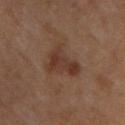biopsy_status: not biopsied; imaged during a skin examination
image:
  source: total-body photography crop
  field_of_view_mm: 15
patient:
  sex: male
  age_approx: 55
automated_metrics:
  cielab_L: 33
  cielab_a: 17
  cielab_b: 24
lesion_size:
  long_diameter_mm_approx: 5.0
site: arm
lighting: cross-polarized